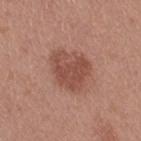| field | value |
|---|---|
| notes | imaged on a skin check; not biopsied |
| diameter | about 5 mm |
| image-analysis metrics | a lesion-detection confidence of about 100/100 |
| location | the right thigh |
| subject | female, about 40 years old |
| imaging modality | ~15 mm tile from a whole-body skin photo |